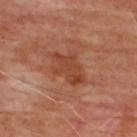diameter: ≈4.5 mm | image: ~15 mm tile from a whole-body skin photo | location: the upper back | subject: male, approximately 65 years of age.